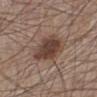{"biopsy_status": "not biopsied; imaged during a skin examination", "lighting": "white-light", "site": "left lower leg", "lesion_size": {"long_diameter_mm_approx": 5.0}, "image": {"source": "total-body photography crop", "field_of_view_mm": 15}, "automated_metrics": {"area_mm2_approx": 14.0, "eccentricity": 0.7, "shape_asymmetry": 0.2, "cielab_L": 41, "cielab_a": 17, "cielab_b": 23, "vs_skin_contrast_norm": 9.5}, "patient": {"sex": "male", "age_approx": 60}}